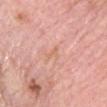{
  "biopsy_status": "not biopsied; imaged during a skin examination",
  "patient": {
    "sex": "male",
    "age_approx": 65
  },
  "lighting": "white-light",
  "site": "front of the torso",
  "image": {
    "source": "total-body photography crop",
    "field_of_view_mm": 15
  }
}